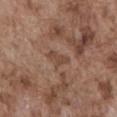follow-up: total-body-photography surveillance lesion; no biopsy
subject: male, in their mid-70s
illumination: white-light illumination
imaging modality: ~15 mm tile from a whole-body skin photo
site: the abdomen
diameter: about 3 mm